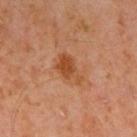Q: Is there a histopathology result?
A: imaged on a skin check; not biopsied
Q: Automated lesion metrics?
A: an area of roughly 6.5 mm², a shape eccentricity near 0.8, and a symmetry-axis asymmetry near 0.4; a border-irregularity rating of about 4/10, a color-variation rating of about 2/10, and a peripheral color-asymmetry measure near 0.5; a classifier nevus-likeness of about 65/100 and a detector confidence of about 100 out of 100 that the crop contains a lesion
Q: What is the anatomic site?
A: the right upper arm
Q: Who is the patient?
A: male, in their 60s
Q: What lighting was used for the tile?
A: cross-polarized
Q: What is the imaging modality?
A: 15 mm crop, total-body photography